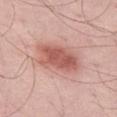The lesion was photographed on a routine skin check and not biopsied; there is no pathology result.